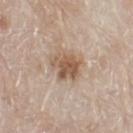Background:
A male patient, roughly 80 years of age. A close-up tile cropped from a whole-body skin photograph, about 15 mm across. The tile uses white-light illumination. From the leg.